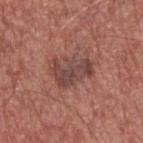| key | value |
|---|---|
| workup | total-body-photography surveillance lesion; no biopsy |
| location | the upper back |
| subject | male, roughly 65 years of age |
| image source | 15 mm crop, total-body photography |
| tile lighting | white-light illumination |
| automated metrics | a lesion color around L≈44 a*≈21 b*≈22 in CIELAB and a lesion–skin lightness drop of about 9; a border-irregularity rating of about 5.5/10, a color-variation rating of about 4/10, and a peripheral color-asymmetry measure near 1 |
| lesion diameter | ≈4.5 mm |